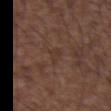Clinical impression: This lesion was catalogued during total-body skin photography and was not selected for biopsy. Image and clinical context: A roughly 15 mm field-of-view crop from a total-body skin photograph. The lesion is located on the upper back. Measured at roughly 3 mm in maximum diameter. A male patient in their 50s.